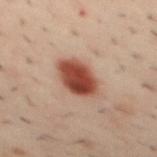The lesion was tiled from a total-body skin photograph and was not biopsied. Cropped from a total-body skin-imaging series; the visible field is about 15 mm. The patient is a male roughly 40 years of age. An algorithmic analysis of the crop reported a shape eccentricity near 0.7 and two-axis asymmetry of about 0.15. And it measured border irregularity of about 1.5 on a 0–10 scale, internal color variation of about 5.5 on a 0–10 scale, and a peripheral color-asymmetry measure near 2. From the mid back. The tile uses cross-polarized illumination. Longest diameter approximately 4.5 mm.